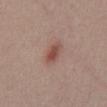Assessment:
Captured during whole-body skin photography for melanoma surveillance; the lesion was not biopsied.
Background:
A 15 mm close-up extracted from a 3D total-body photography capture. From the abdomen. The patient is a male in their mid-50s. Captured under white-light illumination. Approximately 3 mm at its widest.